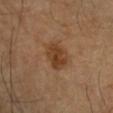biopsy_status: not biopsied; imaged during a skin examination
patient:
  sex: female
  age_approx: 60
automated_metrics:
  area_mm2_approx: 8.0
  shape_asymmetry: 0.15
  nevus_likeness_0_100: 65
  lesion_detection_confidence_0_100: 100
image:
  source: total-body photography crop
  field_of_view_mm: 15
site: right forearm